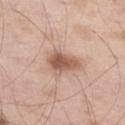Assessment: This lesion was catalogued during total-body skin photography and was not selected for biopsy. Context: The lesion is on the abdomen. Cropped from a whole-body photographic skin survey; the tile spans about 15 mm. Captured under white-light illumination. Approximately 4.5 mm at its widest. The lesion-visualizer software estimated a lesion area of about 10 mm² and an outline eccentricity of about 0.75 (0 = round, 1 = elongated). The software also gave an automated nevus-likeness rating near 95 out of 100. A male subject, about 55 years old.